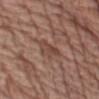Case summary:
- biopsy status — imaged on a skin check; not biopsied
- patient — male, aged approximately 70
- automated metrics — an eccentricity of roughly 0.8 and a shape-asymmetry score of about 0.35 (0 = symmetric); a border-irregularity index near 3.5/10, internal color variation of about 3.5 on a 0–10 scale, and a peripheral color-asymmetry measure near 1.5; a nevus-likeness score of about 0/100 and lesion-presence confidence of about 90/100
- illumination — white-light
- location — the left upper arm
- imaging modality — ~15 mm crop, total-body skin-cancer survey